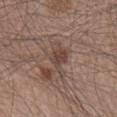follow-up: imaged on a skin check; not biopsied | TBP lesion metrics: an area of roughly 7 mm², an outline eccentricity of about 0.9 (0 = round, 1 = elongated), and a shape-asymmetry score of about 0.4 (0 = symmetric); a border-irregularity rating of about 5/10, internal color variation of about 3 on a 0–10 scale, and peripheral color asymmetry of about 1; an automated nevus-likeness rating near 20 out of 100 and a lesion-detection confidence of about 100/100 | subject: male, aged 43–47 | image source: total-body-photography crop, ~15 mm field of view | illumination: white-light illumination | size: ~5 mm (longest diameter) | body site: the right lower leg.